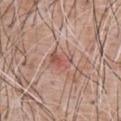Impression:
Captured during whole-body skin photography for melanoma surveillance; the lesion was not biopsied.
Background:
Automated tile analysis of the lesion measured an area of roughly 6 mm², an outline eccentricity of about 0.65 (0 = round, 1 = elongated), and two-axis asymmetry of about 0.2. The software also gave a classifier nevus-likeness of about 0/100 and a lesion-detection confidence of about 100/100. About 3 mm across. The lesion is on the chest. A 15 mm close-up extracted from a 3D total-body photography capture. A male patient approximately 60 years of age. Imaged with white-light lighting.